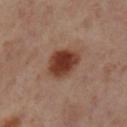follow-up — catalogued during a skin exam; not biopsied
image — 15 mm crop, total-body photography
lighting — cross-polarized illumination
subject — female, aged 53–57
TBP lesion metrics — a footprint of about 11 mm², an outline eccentricity of about 0.65 (0 = round, 1 = elongated), and a symmetry-axis asymmetry near 0.2; roughly 14 lightness units darker than nearby skin and a normalized border contrast of about 12
body site — the left lower leg
lesion diameter — ~4.5 mm (longest diameter)